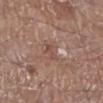Notes:
* notes — total-body-photography surveillance lesion; no biopsy
* patient — male, about 70 years old
* acquisition — total-body-photography crop, ~15 mm field of view
* body site — the right lower leg
* size — ≈2.5 mm